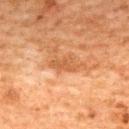follow-up: imaged on a skin check; not biopsied
subject: female, about 60 years old
tile lighting: cross-polarized
image-analysis metrics: an outline eccentricity of about 0.85 (0 = round, 1 = elongated); a border-irregularity rating of about 3/10, a color-variation rating of about 1.5/10, and a peripheral color-asymmetry measure near 0.5; a classifier nevus-likeness of about 0/100 and a detector confidence of about 100 out of 100 that the crop contains a lesion
acquisition: 15 mm crop, total-body photography
anatomic site: the upper back